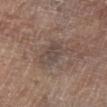This lesion was catalogued during total-body skin photography and was not selected for biopsy. The tile uses cross-polarized illumination. A male patient about 70 years old. A 15 mm close-up extracted from a 3D total-body photography capture. From the right lower leg. Longest diameter approximately 4.5 mm. Automated tile analysis of the lesion measured a symmetry-axis asymmetry near 0.4. The software also gave a lesion-detection confidence of about 95/100.